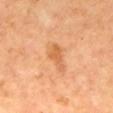The lesion's longest dimension is about 3 mm. This is a cross-polarized tile. The subject is a male aged 63–67. A roughly 15 mm field-of-view crop from a total-body skin photograph. An algorithmic analysis of the crop reported a lesion–skin lightness drop of about 9 and a normalized border contrast of about 6. The analysis additionally found border irregularity of about 4 on a 0–10 scale, a within-lesion color-variation index near 2/10, and peripheral color asymmetry of about 0.5. The software also gave a nevus-likeness score of about 10/100 and lesion-presence confidence of about 100/100. On the mid back.